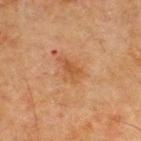Q: Was this lesion biopsied?
A: total-body-photography surveillance lesion; no biopsy
Q: What is the lesion's diameter?
A: ~3.5 mm (longest diameter)
Q: What did automated image analysis measure?
A: a lesion area of about 5 mm², an eccentricity of roughly 0.8, and a shape-asymmetry score of about 0.45 (0 = symmetric); a classifier nevus-likeness of about 0/100 and a lesion-detection confidence of about 100/100
Q: What are the patient's age and sex?
A: male, roughly 70 years of age
Q: What lighting was used for the tile?
A: cross-polarized illumination
Q: Where on the body is the lesion?
A: the upper back
Q: What is the imaging modality?
A: 15 mm crop, total-body photography